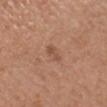The lesion was photographed on a routine skin check and not biopsied; there is no pathology result.
The subject is a female aged 28–32.
This is a white-light tile.
The recorded lesion diameter is about 2.5 mm.
On the head or neck.
An algorithmic analysis of the crop reported border irregularity of about 4.5 on a 0–10 scale and a peripheral color-asymmetry measure near 0.
A 15 mm close-up tile from a total-body photography series done for melanoma screening.